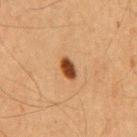The lesion was tiled from a total-body skin photograph and was not biopsied.
Longest diameter approximately 3 mm.
A male patient, roughly 65 years of age.
Imaged with cross-polarized lighting.
A roughly 15 mm field-of-view crop from a total-body skin photograph.
The lesion is located on the chest.